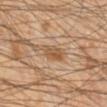Measured at roughly 2.5 mm in maximum diameter. The patient is a male approximately 45 years of age. The lesion is located on the right lower leg. A 15 mm close-up tile from a total-body photography series done for melanoma screening. The tile uses cross-polarized illumination.